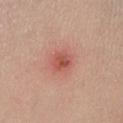Recorded during total-body skin imaging; not selected for excision or biopsy. Imaged with white-light lighting. From the leg. The recorded lesion diameter is about 2.5 mm. The subject is a female aged around 40. A close-up tile cropped from a whole-body skin photograph, about 15 mm across. Automated image analysis of the tile measured internal color variation of about 4 on a 0–10 scale and peripheral color asymmetry of about 1.